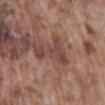site = the lower back | subject = male, aged around 75 | illumination = white-light | image source = ~15 mm crop, total-body skin-cancer survey.